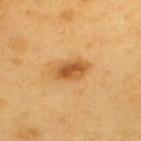The lesion was photographed on a routine skin check and not biopsied; there is no pathology result. Approximately 4 mm at its widest. A male patient, aged 58 to 62. The lesion is on the upper back. This image is a 15 mm lesion crop taken from a total-body photograph. This is a cross-polarized tile.